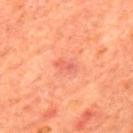Captured during whole-body skin photography for melanoma surveillance; the lesion was not biopsied.
The lesion's longest dimension is about 2.5 mm.
A male subject, in their mid-60s.
A 15 mm close-up extracted from a 3D total-body photography capture.
The total-body-photography lesion software estimated an average lesion color of about L≈57 a*≈33 b*≈33 (CIELAB).
On the mid back.